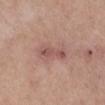Assessment: This lesion was catalogued during total-body skin photography and was not selected for biopsy. Background: The recorded lesion diameter is about 4 mm. Imaged with white-light lighting. A roughly 15 mm field-of-view crop from a total-body skin photograph. On the right lower leg. A female patient, about 55 years old.